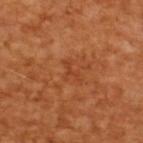This lesion was catalogued during total-body skin photography and was not selected for biopsy.
A region of skin cropped from a whole-body photographic capture, roughly 15 mm wide.
A male patient aged 63–67.
About 3 mm across.
This is a cross-polarized tile.